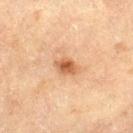The lesion was tiled from a total-body skin photograph and was not biopsied. A male patient, about 70 years old. From the left thigh. About 2.5 mm across. A 15 mm close-up tile from a total-body photography series done for melanoma screening. Captured under cross-polarized illumination.